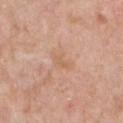No biopsy was performed on this lesion — it was imaged during a full skin examination and was not determined to be concerning. The lesion-visualizer software estimated an automated nevus-likeness rating near 0 out of 100 and lesion-presence confidence of about 100/100. A male subject, aged 58 to 62. Located on the chest. A close-up tile cropped from a whole-body skin photograph, about 15 mm across. Measured at roughly 3 mm in maximum diameter.